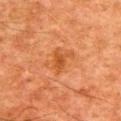Clinical impression:
This lesion was catalogued during total-body skin photography and was not selected for biopsy.
Image and clinical context:
An algorithmic analysis of the crop reported a mean CIELAB color near L≈43 a*≈26 b*≈38, a lesion–skin lightness drop of about 7, and a normalized lesion–skin contrast near 6.5. It also reported border irregularity of about 5.5 on a 0–10 scale, a color-variation rating of about 1.5/10, and radial color variation of about 0.5. The software also gave lesion-presence confidence of about 100/100. About 3 mm across. A 15 mm close-up extracted from a 3D total-body photography capture. Captured under cross-polarized illumination. Located on the upper back. The subject is a male approximately 65 years of age.